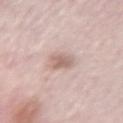The lesion was photographed on a routine skin check and not biopsied; there is no pathology result.
The tile uses white-light illumination.
The lesion's longest dimension is about 3 mm.
A roughly 15 mm field-of-view crop from a total-body skin photograph.
The lesion is located on the left forearm.
A female patient aged around 65.